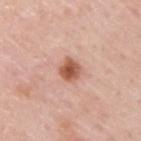| feature | finding |
|---|---|
| biopsy status | imaged on a skin check; not biopsied |
| diameter | about 2.5 mm |
| subject | male, approximately 60 years of age |
| acquisition | total-body-photography crop, ~15 mm field of view |
| location | the upper back |